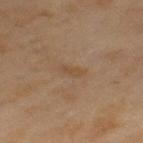Assessment:
Recorded during total-body skin imaging; not selected for excision or biopsy.
Context:
On the left thigh. A 15 mm close-up extracted from a 3D total-body photography capture. The subject is a female aged 58 to 62.